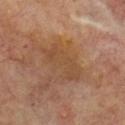Notes:
- subject · male, aged around 70
- lighting · cross-polarized
- lesion diameter · ~6 mm (longest diameter)
- body site · the chest
- TBP lesion metrics · a footprint of about 11 mm², an eccentricity of roughly 0.9, and a symmetry-axis asymmetry near 0.35; a border-irregularity index near 5.5/10 and peripheral color asymmetry of about 0.5; a classifier nevus-likeness of about 0/100 and a detector confidence of about 100 out of 100 that the crop contains a lesion
- acquisition · total-body-photography crop, ~15 mm field of view